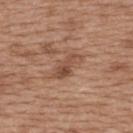{"biopsy_status": "not biopsied; imaged during a skin examination", "image": {"source": "total-body photography crop", "field_of_view_mm": 15}, "automated_metrics": {"area_mm2_approx": 5.5, "eccentricity": 0.9, "lesion_detection_confidence_0_100": 100}, "patient": {"sex": "female", "age_approx": 40}, "lesion_size": {"long_diameter_mm_approx": 4.0}, "lighting": "white-light", "site": "upper back"}